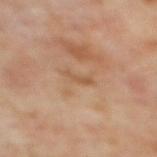Q: Was a biopsy performed?
A: total-body-photography surveillance lesion; no biopsy
Q: Lesion location?
A: the upper back
Q: Who is the patient?
A: female, aged 58–62
Q: How was the tile lit?
A: cross-polarized illumination
Q: Automated lesion metrics?
A: a shape eccentricity near 0.95 and a symmetry-axis asymmetry near 0.45; a nevus-likeness score of about 0/100 and a lesion-detection confidence of about 100/100
Q: How was this image acquired?
A: ~15 mm crop, total-body skin-cancer survey
Q: How large is the lesion?
A: ~3 mm (longest diameter)